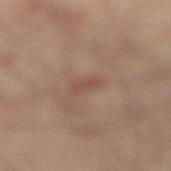The lesion was tiled from a total-body skin photograph and was not biopsied. The lesion is on the left leg. The lesion's longest dimension is about 2.5 mm. A male patient aged 53–57. Cropped from a total-body skin-imaging series; the visible field is about 15 mm.